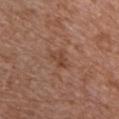Notes:
- patient: male, aged 63 to 67
- imaging modality: 15 mm crop, total-body photography
- site: the chest